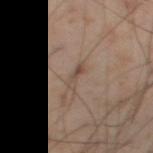Clinical impression:
The lesion was tiled from a total-body skin photograph and was not biopsied.
Clinical summary:
From the right lower leg. A male subject about 55 years old. Cropped from a whole-body photographic skin survey; the tile spans about 15 mm. Captured under cross-polarized illumination.